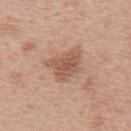No biopsy was performed on this lesion — it was imaged during a full skin examination and was not determined to be concerning. A male subject, approximately 70 years of age. An algorithmic analysis of the crop reported a lesion color around L≈55 a*≈22 b*≈29 in CIELAB and a normalized border contrast of about 7. And it measured an automated nevus-likeness rating near 20 out of 100 and a detector confidence of about 100 out of 100 that the crop contains a lesion. The tile uses white-light illumination. From the mid back. A roughly 15 mm field-of-view crop from a total-body skin photograph. Longest diameter approximately 4 mm.